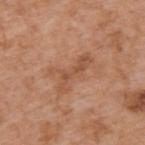Clinical impression: The lesion was tiled from a total-body skin photograph and was not biopsied. Acquisition and patient details: Measured at roughly 5 mm in maximum diameter. The lesion is located on the back. The lesion-visualizer software estimated a footprint of about 7 mm² and a shape-asymmetry score of about 0.65 (0 = symmetric). It also reported about 8 CIELAB-L* units darker than the surrounding skin and a normalized lesion–skin contrast near 6. The analysis additionally found a border-irregularity rating of about 10/10, internal color variation of about 1 on a 0–10 scale, and peripheral color asymmetry of about 0.5. It also reported an automated nevus-likeness rating near 0 out of 100 and a detector confidence of about 100 out of 100 that the crop contains a lesion. A male patient roughly 65 years of age. Cropped from a whole-body photographic skin survey; the tile spans about 15 mm.